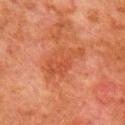Part of a total-body skin-imaging series; this lesion was reviewed on a skin check and was not flagged for biopsy. The lesion is on the right upper arm. This image is a 15 mm lesion crop taken from a total-body photograph. A male subject aged 78–82. About 5.5 mm across. The lesion-visualizer software estimated a lesion–skin lightness drop of about 6. And it measured a detector confidence of about 100 out of 100 that the crop contains a lesion.